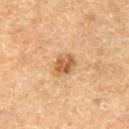Q: Is there a histopathology result?
A: no biopsy performed (imaged during a skin exam)
Q: Lesion size?
A: ~3.5 mm (longest diameter)
Q: What lighting was used for the tile?
A: cross-polarized illumination
Q: Automated lesion metrics?
A: a lesion–skin lightness drop of about 10 and a lesion-to-skin contrast of about 7.5 (normalized; higher = more distinct); internal color variation of about 3.5 on a 0–10 scale and a peripheral color-asymmetry measure near 1.5; a classifier nevus-likeness of about 85/100 and a lesion-detection confidence of about 100/100
Q: What is the anatomic site?
A: the left thigh
Q: Who is the patient?
A: male, roughly 75 years of age
Q: How was this image acquired?
A: total-body-photography crop, ~15 mm field of view